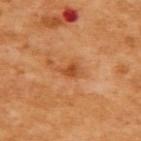Recorded during total-body skin imaging; not selected for excision or biopsy. A female patient, approximately 55 years of age. A close-up tile cropped from a whole-body skin photograph, about 15 mm across. The lesion-visualizer software estimated a footprint of about 3.5 mm² and an outline eccentricity of about 0.75 (0 = round, 1 = elongated). It also reported border irregularity of about 4 on a 0–10 scale and internal color variation of about 2 on a 0–10 scale. The lesion is on the upper back. Captured under cross-polarized illumination.